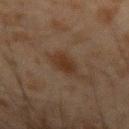No biopsy was performed on this lesion — it was imaged during a full skin examination and was not determined to be concerning. From the left forearm. Longest diameter approximately 3.5 mm. A male patient, in their mid-40s. A close-up tile cropped from a whole-body skin photograph, about 15 mm across.